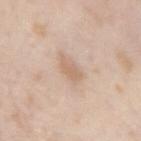Clinical impression: The lesion was photographed on a routine skin check and not biopsied; there is no pathology result. Background: The lesion is on the right forearm. Automated tile analysis of the lesion measured an area of roughly 3.5 mm² and a symmetry-axis asymmetry near 0.45. The analysis additionally found a lesion color around L≈65 a*≈17 b*≈30 in CIELAB, roughly 8 lightness units darker than nearby skin, and a normalized lesion–skin contrast near 5.5. A male subject, aged 43 to 47. The tile uses white-light illumination. Cropped from a whole-body photographic skin survey; the tile spans about 15 mm. About 3 mm across.